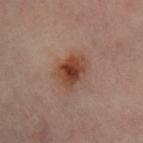Imaged during a routine full-body skin examination; the lesion was not biopsied and no histopathology is available. Approximately 4 mm at its widest. A 15 mm close-up extracted from a 3D total-body photography capture. From the left leg. A female subject about 60 years old. Captured under cross-polarized illumination. An algorithmic analysis of the crop reported a border-irregularity index near 2/10, internal color variation of about 5.5 on a 0–10 scale, and a peripheral color-asymmetry measure near 1. It also reported an automated nevus-likeness rating near 95 out of 100 and lesion-presence confidence of about 100/100.